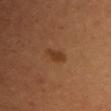Recorded during total-body skin imaging; not selected for excision or biopsy. Imaged with cross-polarized lighting. A roughly 15 mm field-of-view crop from a total-body skin photograph. About 2.5 mm across. Automated image analysis of the tile measured a footprint of about 3 mm², an outline eccentricity of about 0.85 (0 = round, 1 = elongated), and a symmetry-axis asymmetry near 0.4. The software also gave a lesion color around L≈38 a*≈23 b*≈35 in CIELAB, roughly 8 lightness units darker than nearby skin, and a normalized lesion–skin contrast near 7.5. It also reported internal color variation of about 1 on a 0–10 scale and a peripheral color-asymmetry measure near 0.5. It also reported a classifier nevus-likeness of about 90/100 and a lesion-detection confidence of about 100/100. The patient is a female about 50 years old. The lesion is on the chest.